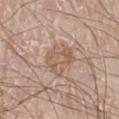Q: Was a biopsy performed?
A: total-body-photography surveillance lesion; no biopsy
Q: Where on the body is the lesion?
A: the left leg
Q: What are the patient's age and sex?
A: male, aged approximately 80
Q: What kind of image is this?
A: 15 mm crop, total-body photography